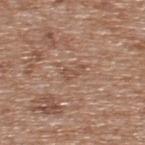| field | value |
|---|---|
| biopsy status | catalogued during a skin exam; not biopsied |
| lighting | white-light |
| automated lesion analysis | a lesion area of about 2.5 mm², a shape eccentricity near 0.9, and a symmetry-axis asymmetry near 0.45; a classifier nevus-likeness of about 0/100 |
| lesion size | ≈2.5 mm |
| image | ~15 mm crop, total-body skin-cancer survey |
| subject | male, in their mid- to late 60s |
| site | the upper back |